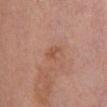workup = no biopsy performed (imaged during a skin exam); patient = female, aged 63–67; image = total-body-photography crop, ~15 mm field of view; site = the chest.